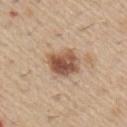follow-up: total-body-photography surveillance lesion; no biopsy
site: the right upper arm
imaging modality: ~15 mm crop, total-body skin-cancer survey
tile lighting: white-light illumination
lesion size: ≈4 mm
patient: male, in their 60s
automated lesion analysis: an average lesion color of about L≈54 a*≈20 b*≈30 (CIELAB), about 15 CIELAB-L* units darker than the surrounding skin, and a normalized border contrast of about 9.5; internal color variation of about 7 on a 0–10 scale and radial color variation of about 2.5